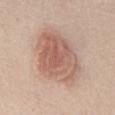Imaged during a routine full-body skin examination; the lesion was not biopsied and no histopathology is available. A female patient roughly 40 years of age. The lesion is located on the chest. A lesion tile, about 15 mm wide, cut from a 3D total-body photograph. Automated image analysis of the tile measured a lesion area of about 28 mm², a shape eccentricity near 0.65, and a shape-asymmetry score of about 0.2 (0 = symmetric). The software also gave border irregularity of about 2.5 on a 0–10 scale, a within-lesion color-variation index near 5/10, and radial color variation of about 1.5. The tile uses white-light illumination. Measured at roughly 7 mm in maximum diameter.